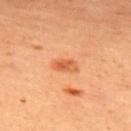Q: Was a biopsy performed?
A: catalogued during a skin exam; not biopsied
Q: Lesion location?
A: the upper back
Q: What lighting was used for the tile?
A: cross-polarized illumination
Q: What kind of image is this?
A: 15 mm crop, total-body photography
Q: Patient demographics?
A: female, aged approximately 35